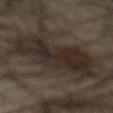Longest diameter approximately 8 mm. A male patient, in their mid-60s. Captured under cross-polarized illumination. On the abdomen. An algorithmic analysis of the crop reported a lesion color around L≈20 a*≈8 b*≈14 in CIELAB and a normalized lesion–skin contrast near 8.5. It also reported internal color variation of about 4 on a 0–10 scale and peripheral color asymmetry of about 1.5. It also reported a classifier nevus-likeness of about 5/100 and a detector confidence of about 95 out of 100 that the crop contains a lesion. A 15 mm close-up tile from a total-body photography series done for melanoma screening.